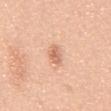Impression:
The lesion was tiled from a total-body skin photograph and was not biopsied.
Clinical summary:
A lesion tile, about 15 mm wide, cut from a 3D total-body photograph. A male patient, approximately 50 years of age. The tile uses white-light illumination. Approximately 3 mm at its widest. From the abdomen.